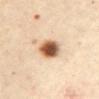biopsy status: catalogued during a skin exam; not biopsied
body site: the abdomen
subject: female, in their 60s
acquisition: 15 mm crop, total-body photography